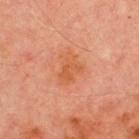| key | value |
|---|---|
| image | ~15 mm crop, total-body skin-cancer survey |
| illumination | cross-polarized illumination |
| patient | male, aged 68–72 |
| image-analysis metrics | a shape eccentricity near 0.7 and a shape-asymmetry score of about 0.4 (0 = symmetric); an average lesion color of about L≈47 a*≈25 b*≈34 (CIELAB) and a lesion–skin lightness drop of about 6; a border-irregularity index near 4.5/10 and a peripheral color-asymmetry measure near 1 |
| site | the chest |
| lesion diameter | ~4 mm (longest diameter) |